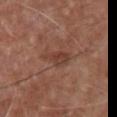Captured during whole-body skin photography for melanoma surveillance; the lesion was not biopsied.
A roughly 15 mm field-of-view crop from a total-body skin photograph.
A male subject, aged around 65.
Measured at roughly 3.5 mm in maximum diameter.
The tile uses white-light illumination.
The lesion is on the chest.